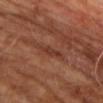– image — ~15 mm tile from a whole-body skin photo
– size — about 3 mm
– subject — male, aged around 60
– body site — the upper back